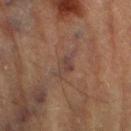biopsy status: total-body-photography surveillance lesion; no biopsy | patient: male, aged 83 or older | lesion diameter: about 3.5 mm | image-analysis metrics: an average lesion color of about L≈35 a*≈15 b*≈20 (CIELAB), roughly 5 lightness units darker than nearby skin, and a lesion-to-skin contrast of about 6 (normalized; higher = more distinct); border irregularity of about 4 on a 0–10 scale and a within-lesion color-variation index near 2/10; an automated nevus-likeness rating near 0 out of 100 | body site: the left lower leg | image: total-body-photography crop, ~15 mm field of view | illumination: cross-polarized.